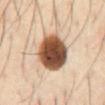Q: Is there a histopathology result?
A: catalogued during a skin exam; not biopsied
Q: What is the anatomic site?
A: the chest
Q: Lesion size?
A: about 6 mm
Q: Patient demographics?
A: male, aged approximately 40
Q: Illumination type?
A: cross-polarized
Q: What is the imaging modality?
A: 15 mm crop, total-body photography